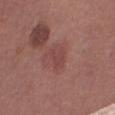This lesion was catalogued during total-body skin photography and was not selected for biopsy.
A female patient aged around 40.
The lesion is on the right thigh.
A 15 mm crop from a total-body photograph taken for skin-cancer surveillance.